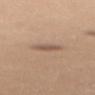No biopsy was performed on this lesion — it was imaged during a full skin examination and was not determined to be concerning. The total-body-photography lesion software estimated a footprint of about 3.5 mm², an outline eccentricity of about 0.75 (0 = round, 1 = elongated), and two-axis asymmetry of about 0.25. From the upper back. A female patient about 30 years old. Measured at roughly 2.5 mm in maximum diameter. The tile uses white-light illumination. A 15 mm crop from a total-body photograph taken for skin-cancer surveillance.